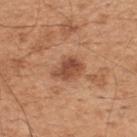Captured during whole-body skin photography for melanoma surveillance; the lesion was not biopsied.
The lesion's longest dimension is about 3.5 mm.
A 15 mm crop from a total-body photograph taken for skin-cancer surveillance.
The lesion is located on the upper back.
A male subject, aged approximately 45.
Imaged with white-light lighting.